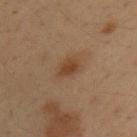biopsy_status: not biopsied; imaged during a skin examination
lighting: cross-polarized
site: left upper arm
lesion_size:
  long_diameter_mm_approx: 3.0
image:
  source: total-body photography crop
  field_of_view_mm: 15
patient:
  sex: male
  age_approx: 35
automated_metrics:
  cielab_L: 38
  cielab_a: 18
  cielab_b: 29
  vs_skin_darker_L: 8.0
  border_irregularity_0_10: 2.5
  peripheral_color_asymmetry: 0.5
  nevus_likeness_0_100: 90
  lesion_detection_confidence_0_100: 100